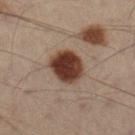No biopsy was performed on this lesion — it was imaged during a full skin examination and was not determined to be concerning. The lesion is on the right lower leg. The patient is a male about 55 years old. The recorded lesion diameter is about 4 mm. A 15 mm close-up extracted from a 3D total-body photography capture. The tile uses cross-polarized illumination.